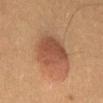{"biopsy_status": "not biopsied; imaged during a skin examination", "patient": {"sex": "male", "age_approx": 50}, "lighting": "cross-polarized", "lesion_size": {"long_diameter_mm_approx": 4.5}, "image": {"source": "total-body photography crop", "field_of_view_mm": 15}, "site": "abdomen"}